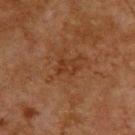{
  "biopsy_status": "not biopsied; imaged during a skin examination",
  "image": {
    "source": "total-body photography crop",
    "field_of_view_mm": 15
  },
  "site": "back",
  "lesion_size": {
    "long_diameter_mm_approx": 3.0
  },
  "patient": {
    "sex": "male",
    "age_approx": 65
  },
  "automated_metrics": {
    "area_mm2_approx": 4.0,
    "shape_asymmetry": 0.35,
    "cielab_L": 28,
    "cielab_a": 19,
    "cielab_b": 28,
    "vs_skin_darker_L": 5.0,
    "vs_skin_contrast_norm": 5.5,
    "border_irregularity_0_10": 4.0,
    "color_variation_0_10": 2.5,
    "peripheral_color_asymmetry": 1.0,
    "nevus_likeness_0_100": 0,
    "lesion_detection_confidence_0_100": 100
  },
  "lighting": "cross-polarized"
}